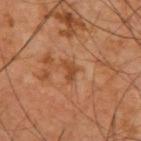Imaged during a routine full-body skin examination; the lesion was not biopsied and no histopathology is available. Longest diameter approximately 2.5 mm. A male patient approximately 60 years of age. A 15 mm crop from a total-body photograph taken for skin-cancer surveillance. On the upper back. The tile uses cross-polarized illumination.